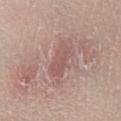Q: Was a biopsy performed?
A: no biopsy performed (imaged during a skin exam)
Q: How large is the lesion?
A: ~5 mm (longest diameter)
Q: Lesion location?
A: the left lower leg
Q: Who is the patient?
A: male, aged approximately 35
Q: What kind of image is this?
A: 15 mm crop, total-body photography